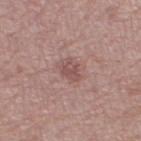Impression:
This lesion was catalogued during total-body skin photography and was not selected for biopsy.
Image and clinical context:
A roughly 15 mm field-of-view crop from a total-body skin photograph. The lesion is located on the left thigh. Longest diameter approximately 2.5 mm. Captured under white-light illumination. A female patient, aged 63 to 67.